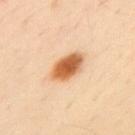Q: Is there a histopathology result?
A: total-body-photography surveillance lesion; no biopsy
Q: Who is the patient?
A: male, roughly 50 years of age
Q: How large is the lesion?
A: ≈4.5 mm
Q: What is the anatomic site?
A: the upper back
Q: What lighting was used for the tile?
A: cross-polarized
Q: How was this image acquired?
A: ~15 mm tile from a whole-body skin photo